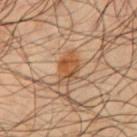{"biopsy_status": "not biopsied; imaged during a skin examination", "site": "chest", "automated_metrics": {"cielab_L": 52, "cielab_a": 22, "cielab_b": 37, "vs_skin_darker_L": 10.0, "border_irregularity_0_10": 3.0, "color_variation_0_10": 5.0, "peripheral_color_asymmetry": 1.5, "nevus_likeness_0_100": 85}, "lesion_size": {"long_diameter_mm_approx": 3.5}, "patient": {"sex": "male", "age_approx": 65}, "lighting": "cross-polarized", "image": {"source": "total-body photography crop", "field_of_view_mm": 15}}